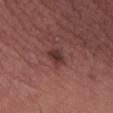biopsy_status: not biopsied; imaged during a skin examination
patient:
  sex: female
  age_approx: 30
image:
  source: total-body photography crop
  field_of_view_mm: 15
site: abdomen
lighting: white-light
lesion_size:
  long_diameter_mm_approx: 2.5